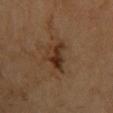This lesion was catalogued during total-body skin photography and was not selected for biopsy. The lesion is located on the chest. The patient is a male about 70 years old. Automated tile analysis of the lesion measured border irregularity of about 5 on a 0–10 scale, internal color variation of about 6.5 on a 0–10 scale, and a peripheral color-asymmetry measure near 2.5. It also reported a classifier nevus-likeness of about 45/100 and lesion-presence confidence of about 100/100. This is a cross-polarized tile. Longest diameter approximately 4 mm. A 15 mm close-up tile from a total-body photography series done for melanoma screening.